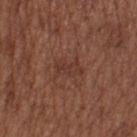Recorded during total-body skin imaging; not selected for excision or biopsy.
The lesion is located on the leg.
A female subject, aged 53 to 57.
Imaged with white-light lighting.
The recorded lesion diameter is about 3 mm.
A 15 mm close-up tile from a total-body photography series done for melanoma screening.